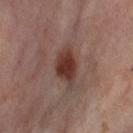Case summary:
• workup · catalogued during a skin exam; not biopsied
• lesion diameter · ≈5 mm
• acquisition · ~15 mm crop, total-body skin-cancer survey
• patient · female, aged 53–57
• location · the right thigh
• lighting · cross-polarized illumination